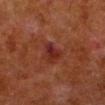The lesion was photographed on a routine skin check and not biopsied; there is no pathology result.
On the left lower leg.
A male subject about 80 years old.
A 15 mm close-up tile from a total-body photography series done for melanoma screening.